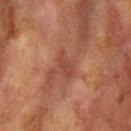This lesion was catalogued during total-body skin photography and was not selected for biopsy. A female subject aged 73 to 77. A region of skin cropped from a whole-body photographic capture, roughly 15 mm wide. On the right upper arm. Approximately 3.5 mm at its widest. The tile uses cross-polarized illumination.